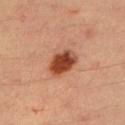– follow-up · imaged on a skin check; not biopsied
– lesion size · ≈4 mm
– location · the left upper arm
– tile lighting · cross-polarized illumination
– acquisition · 15 mm crop, total-body photography
– subject · male, aged 33 to 37